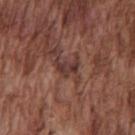Q: Lesion location?
A: the chest
Q: How was this image acquired?
A: total-body-photography crop, ~15 mm field of view
Q: Illumination type?
A: white-light
Q: What are the patient's age and sex?
A: male, in their mid- to late 70s
Q: How large is the lesion?
A: ~2.5 mm (longest diameter)
Q: Automated lesion metrics?
A: a lesion color around L≈35 a*≈21 b*≈22 in CIELAB, about 9 CIELAB-L* units darker than the surrounding skin, and a lesion-to-skin contrast of about 8.5 (normalized; higher = more distinct)